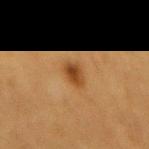<lesion>
  <biopsy_status>not biopsied; imaged during a skin examination</biopsy_status>
  <image>
    <source>total-body photography crop</source>
    <field_of_view_mm>15</field_of_view_mm>
  </image>
  <lesion_size>
    <long_diameter_mm_approx>3.0</long_diameter_mm_approx>
  </lesion_size>
  <automated_metrics>
    <area_mm2_approx>4.5</area_mm2_approx>
    <shape_asymmetry>0.2</shape_asymmetry>
    <border_irregularity_0_10>2.0</border_irregularity_0_10>
    <color_variation_0_10>3.5</color_variation_0_10>
    <peripheral_color_asymmetry>1.0</peripheral_color_asymmetry>
  </automated_metrics>
  <patient>
    <sex>female</sex>
    <age_approx>55</age_approx>
  </patient>
  <site>mid back</site>
  <lighting>cross-polarized</lighting>
</lesion>